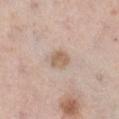Q: Is there a histopathology result?
A: catalogued during a skin exam; not biopsied
Q: Illumination type?
A: white-light
Q: What are the patient's age and sex?
A: female, in their mid-60s
Q: Lesion location?
A: the right lower leg
Q: What kind of image is this?
A: 15 mm crop, total-body photography
Q: What did automated image analysis measure?
A: an outline eccentricity of about 0.6 (0 = round, 1 = elongated) and two-axis asymmetry of about 0.15; a classifier nevus-likeness of about 30/100 and a detector confidence of about 100 out of 100 that the crop contains a lesion